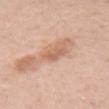Q: How large is the lesion?
A: about 2.5 mm
Q: Who is the patient?
A: female, aged approximately 30
Q: What is the anatomic site?
A: the right upper arm
Q: What is the imaging modality?
A: ~15 mm crop, total-body skin-cancer survey
Q: What did automated image analysis measure?
A: a mean CIELAB color near L≈62 a*≈22 b*≈31, about 8 CIELAB-L* units darker than the surrounding skin, and a normalized lesion–skin contrast near 5.5; border irregularity of about 2.5 on a 0–10 scale and a within-lesion color-variation index near 1.5/10